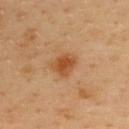workup = no biopsy performed (imaged during a skin exam)
imaging modality = ~15 mm tile from a whole-body skin photo
site = the upper back
lesion size = ≈3 mm
patient = male, approximately 40 years of age
lighting = cross-polarized